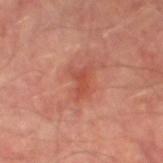The lesion was photographed on a routine skin check and not biopsied; there is no pathology result.
The lesion is located on the left thigh.
The lesion's longest dimension is about 3.5 mm.
A close-up tile cropped from a whole-body skin photograph, about 15 mm across.
Imaged with cross-polarized lighting.
A male patient aged around 65.